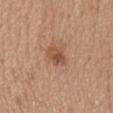follow-up = no biopsy performed (imaged during a skin exam); site = the head or neck; lesion diameter = ~3 mm (longest diameter); acquisition = total-body-photography crop, ~15 mm field of view; tile lighting = white-light illumination; patient = female, approximately 35 years of age.